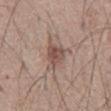Impression:
No biopsy was performed on this lesion — it was imaged during a full skin examination and was not determined to be concerning.
Acquisition and patient details:
A 15 mm close-up tile from a total-body photography series done for melanoma screening. From the abdomen. Approximately 3.5 mm at its widest. The tile uses white-light illumination. A male patient, aged approximately 65.